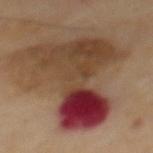Imaged during a routine full-body skin examination; the lesion was not biopsied and no histopathology is available.
Imaged with cross-polarized lighting.
The total-body-photography lesion software estimated an area of roughly 50 mm², a shape eccentricity near 0.75, and two-axis asymmetry of about 0.6. And it measured a nevus-likeness score of about 0/100 and lesion-presence confidence of about 100/100.
The lesion is on the mid back.
A male subject roughly 70 years of age.
Measured at roughly 11 mm in maximum diameter.
Cropped from a total-body skin-imaging series; the visible field is about 15 mm.